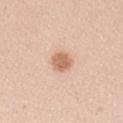| feature | finding |
|---|---|
| workup | no biopsy performed (imaged during a skin exam) |
| image | total-body-photography crop, ~15 mm field of view |
| size | about 3 mm |
| subject | female, aged 23–27 |
| site | the arm |
| tile lighting | white-light |
| automated lesion analysis | a lesion area of about 5.5 mm², an eccentricity of roughly 0.65, and a shape-asymmetry score of about 0.2 (0 = symmetric); a lesion color around L≈65 a*≈21 b*≈32 in CIELAB and a lesion–skin lightness drop of about 12; a nevus-likeness score of about 95/100 and lesion-presence confidence of about 100/100 |